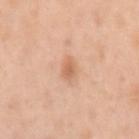The lesion was photographed on a routine skin check and not biopsied; there is no pathology result.
Cropped from a whole-body photographic skin survey; the tile spans about 15 mm.
The patient is a female aged approximately 45.
On the left upper arm.